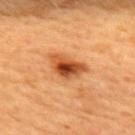biopsy_status: not biopsied; imaged during a skin examination
image:
  source: total-body photography crop
  field_of_view_mm: 15
lesion_size:
  long_diameter_mm_approx: 4.5
site: upper back
automated_metrics:
  eccentricity: 0.8
  cielab_L: 52
  cielab_a: 30
  cielab_b: 43
  vs_skin_darker_L: 16.0
  vs_skin_contrast_norm: 10.5
  border_irregularity_0_10: 3.0
  color_variation_0_10: 9.5
  peripheral_color_asymmetry: 3.0
  lesion_detection_confidence_0_100: 100
lighting: cross-polarized
patient:
  sex: female
  age_approx: 45